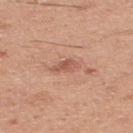| key | value |
|---|---|
| follow-up | imaged on a skin check; not biopsied |
| diameter | ~3 mm (longest diameter) |
| subject | male, roughly 45 years of age |
| imaging modality | 15 mm crop, total-body photography |
| anatomic site | the upper back |
| lighting | white-light illumination |